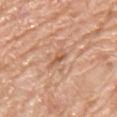follow-up = no biopsy performed (imaged during a skin exam); patient = male, in their 60s; location = the back; imaging modality = 15 mm crop, total-body photography.